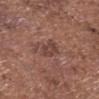| key | value |
|---|---|
| workup | imaged on a skin check; not biopsied |
| lighting | white-light illumination |
| body site | the head or neck |
| lesion size | ~3.5 mm (longest diameter) |
| image source | ~15 mm tile from a whole-body skin photo |
| TBP lesion metrics | a border-irregularity rating of about 3.5/10, a within-lesion color-variation index near 1.5/10, and radial color variation of about 0.5; an automated nevus-likeness rating near 0 out of 100 |
| subject | male, roughly 75 years of age |